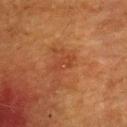Clinical impression:
Imaged during a routine full-body skin examination; the lesion was not biopsied and no histopathology is available.